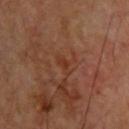{"patient": {"sex": "male", "age_approx": 60}, "lesion_size": {"long_diameter_mm_approx": 2.5}, "site": "upper back", "image": {"source": "total-body photography crop", "field_of_view_mm": 15}, "lighting": "cross-polarized"}